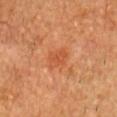Captured during whole-body skin photography for melanoma surveillance; the lesion was not biopsied.
Longest diameter approximately 3 mm.
A male subject, aged 83 or older.
Imaged with cross-polarized lighting.
Located on the head or neck.
This image is a 15 mm lesion crop taken from a total-body photograph.